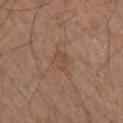Assessment: Imaged during a routine full-body skin examination; the lesion was not biopsied and no histopathology is available. Image and clinical context: The tile uses white-light illumination. A male patient, aged 53 to 57. Cropped from a whole-body photographic skin survey; the tile spans about 15 mm. On the left forearm. Approximately 3 mm at its widest.